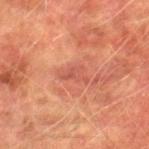{
  "biopsy_status": "not biopsied; imaged during a skin examination"
}